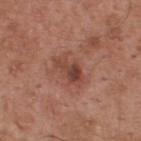Impression:
Captured during whole-body skin photography for melanoma surveillance; the lesion was not biopsied.
Context:
The lesion is located on the back. A 15 mm crop from a total-body photograph taken for skin-cancer surveillance. A male patient aged 53 to 57. Captured under white-light illumination.